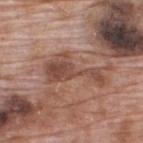Part of a total-body skin-imaging series; this lesion was reviewed on a skin check and was not flagged for biopsy.
The patient is a male roughly 70 years of age.
A 15 mm close-up extracted from a 3D total-body photography capture.
Captured under white-light illumination.
Located on the upper back.
An algorithmic analysis of the crop reported an area of roughly 14 mm² and an eccentricity of roughly 0.9. It also reported a lesion color around L≈48 a*≈21 b*≈26 in CIELAB, roughly 10 lightness units darker than nearby skin, and a lesion-to-skin contrast of about 7 (normalized; higher = more distinct). The software also gave border irregularity of about 8.5 on a 0–10 scale and a peripheral color-asymmetry measure near 1. The analysis additionally found a classifier nevus-likeness of about 0/100 and lesion-presence confidence of about 95/100.
Approximately 7 mm at its widest.